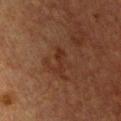Clinical summary: A female patient, in their 40s. Measured at roughly 3.5 mm in maximum diameter. This is a cross-polarized tile. A 15 mm close-up tile from a total-body photography series done for melanoma screening. Located on the chest.